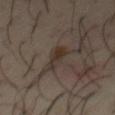Captured during whole-body skin photography for melanoma surveillance; the lesion was not biopsied.
A male patient aged 38–42.
Cropped from a whole-body photographic skin survey; the tile spans about 15 mm.
The tile uses cross-polarized illumination.
The lesion-visualizer software estimated a border-irregularity rating of about 4/10, a color-variation rating of about 3/10, and a peripheral color-asymmetry measure near 1.
Located on the chest.
Longest diameter approximately 4.5 mm.